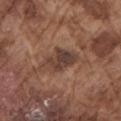workup: no biopsy performed (imaged during a skin exam)
subject: male, aged approximately 75
lesion diameter: about 4.5 mm
automated lesion analysis: a footprint of about 8.5 mm² and an outline eccentricity of about 0.8 (0 = round, 1 = elongated); a mean CIELAB color near L≈39 a*≈18 b*≈24, roughly 10 lightness units darker than nearby skin, and a normalized lesion–skin contrast near 9; a border-irregularity index near 3.5/10, a within-lesion color-variation index near 5.5/10, and radial color variation of about 2
location: the left upper arm
tile lighting: white-light illumination
image source: ~15 mm crop, total-body skin-cancer survey